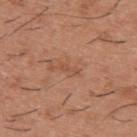Assessment:
Recorded during total-body skin imaging; not selected for excision or biopsy.
Background:
A male subject, approximately 40 years of age. Located on the upper back. Imaged with white-light lighting. Longest diameter approximately 2.5 mm. A 15 mm close-up tile from a total-body photography series done for melanoma screening.